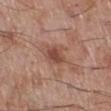Notes:
– workup — no biopsy performed (imaged during a skin exam)
– automated lesion analysis — a lesion area of about 5 mm² and a shape-asymmetry score of about 0.3 (0 = symmetric)
– diameter — ~2.5 mm (longest diameter)
– patient — male, approximately 70 years of age
– location — the right lower leg
– imaging modality — ~15 mm tile from a whole-body skin photo